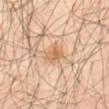Imaged during a routine full-body skin examination; the lesion was not biopsied and no histopathology is available.
Imaged with cross-polarized lighting.
Measured at roughly 2.5 mm in maximum diameter.
From the abdomen.
Cropped from a whole-body photographic skin survey; the tile spans about 15 mm.
An algorithmic analysis of the crop reported a mean CIELAB color near L≈63 a*≈19 b*≈36 and a lesion–skin lightness drop of about 8. And it measured an automated nevus-likeness rating near 75 out of 100 and lesion-presence confidence of about 100/100.
A subject about 65 years old.